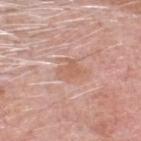Case summary:
– diameter · ≈3.5 mm
– acquisition · ~15 mm tile from a whole-body skin photo
– subject · male, about 70 years old
– TBP lesion metrics · a lesion area of about 5.5 mm² and an outline eccentricity of about 0.65 (0 = round, 1 = elongated); a color-variation rating of about 1.5/10 and peripheral color asymmetry of about 0.5; a classifier nevus-likeness of about 0/100 and a detector confidence of about 100 out of 100 that the crop contains a lesion
– site · the head or neck
– illumination · white-light illumination